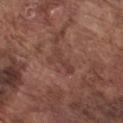follow-up: imaged on a skin check; not biopsied | lighting: white-light | patient: male, about 75 years old | image-analysis metrics: a footprint of about 4.5 mm², an outline eccentricity of about 0.9 (0 = round, 1 = elongated), and a shape-asymmetry score of about 0.6 (0 = symmetric); a mean CIELAB color near L≈40 a*≈21 b*≈23, a lesion–skin lightness drop of about 6, and a normalized border contrast of about 5.5; a border-irregularity rating of about 7.5/10 and a within-lesion color-variation index near 0/10; a nevus-likeness score of about 0/100 and lesion-presence confidence of about 65/100 | lesion diameter: about 4 mm | site: the chest | acquisition: ~15 mm crop, total-body skin-cancer survey.